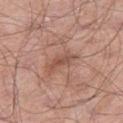<tbp_lesion>
  <biopsy_status>not biopsied; imaged during a skin examination</biopsy_status>
  <patient>
    <sex>male</sex>
    <age_approx>70</age_approx>
  </patient>
  <image>
    <source>total-body photography crop</source>
    <field_of_view_mm>15</field_of_view_mm>
  </image>
  <lesion_size>
    <long_diameter_mm_approx>4.0</long_diameter_mm_approx>
  </lesion_size>
  <site>leg</site>
  <lighting>white-light</lighting>
</tbp_lesion>